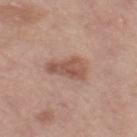notes: imaged on a skin check; not biopsied.